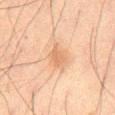No biopsy was performed on this lesion — it was imaged during a full skin examination and was not determined to be concerning.
The lesion's longest dimension is about 2.5 mm.
An algorithmic analysis of the crop reported a border-irregularity rating of about 3/10 and peripheral color asymmetry of about 0.5. It also reported a classifier nevus-likeness of about 15/100.
Captured under cross-polarized illumination.
Located on the lower back.
Cropped from a whole-body photographic skin survey; the tile spans about 15 mm.
The subject is a male aged 63–67.